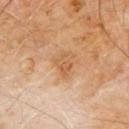Part of a total-body skin-imaging series; this lesion was reviewed on a skin check and was not flagged for biopsy.
The lesion-visualizer software estimated an average lesion color of about L≈56 a*≈21 b*≈37 (CIELAB), roughly 8 lightness units darker than nearby skin, and a lesion-to-skin contrast of about 6 (normalized; higher = more distinct). And it measured a border-irregularity index near 4/10 and a within-lesion color-variation index near 2/10.
The tile uses cross-polarized illumination.
On the chest.
A 15 mm crop from a total-body photograph taken for skin-cancer surveillance.
The subject is a male approximately 60 years of age.
Measured at roughly 2.5 mm in maximum diameter.